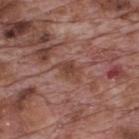workup = total-body-photography surveillance lesion; no biopsy
diameter = ~2.5 mm (longest diameter)
image source = ~15 mm crop, total-body skin-cancer survey
TBP lesion metrics = a lesion area of about 4.5 mm², a shape eccentricity near 0.75, and two-axis asymmetry of about 0.25; a lesion–skin lightness drop of about 8 and a normalized lesion–skin contrast near 6.5; an automated nevus-likeness rating near 0 out of 100
subject = male, roughly 70 years of age
body site = the upper back